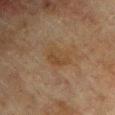workup: imaged on a skin check; not biopsied
patient: male, aged approximately 75
tile lighting: cross-polarized
location: the left upper arm
TBP lesion metrics: two-axis asymmetry of about 0.25; an average lesion color of about L≈36 a*≈14 b*≈28 (CIELAB) and a lesion-to-skin contrast of about 5.5 (normalized; higher = more distinct)
acquisition: ~15 mm tile from a whole-body skin photo